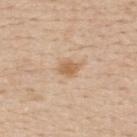Imaged during a routine full-body skin examination; the lesion was not biopsied and no histopathology is available. The lesion-visualizer software estimated an outline eccentricity of about 0.7 (0 = round, 1 = elongated). It also reported a lesion color around L≈62 a*≈18 b*≈35 in CIELAB and a lesion–skin lightness drop of about 10. And it measured a border-irregularity index near 2.5/10, a color-variation rating of about 1.5/10, and radial color variation of about 0.5. A lesion tile, about 15 mm wide, cut from a 3D total-body photograph. Imaged with white-light lighting. The lesion is located on the back. Measured at roughly 2.5 mm in maximum diameter. A female subject, in their mid- to late 50s.